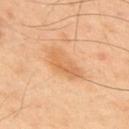Imaged during a routine full-body skin examination; the lesion was not biopsied and no histopathology is available.
Measured at roughly 4.5 mm in maximum diameter.
On the upper back.
An algorithmic analysis of the crop reported a lesion area of about 8.5 mm², an outline eccentricity of about 0.9 (0 = round, 1 = elongated), and a shape-asymmetry score of about 0.35 (0 = symmetric). It also reported a mean CIELAB color near L≈64 a*≈23 b*≈41, roughly 8 lightness units darker than nearby skin, and a lesion-to-skin contrast of about 6 (normalized; higher = more distinct). The software also gave border irregularity of about 4 on a 0–10 scale, a color-variation rating of about 2.5/10, and a peripheral color-asymmetry measure near 1.
A roughly 15 mm field-of-view crop from a total-body skin photograph.
A male subject aged around 50.
This is a cross-polarized tile.